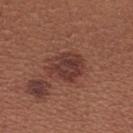Imaged during a routine full-body skin examination; the lesion was not biopsied and no histopathology is available.
Located on the upper back.
About 4.5 mm across.
A lesion tile, about 15 mm wide, cut from a 3D total-body photograph.
A female patient, roughly 25 years of age.